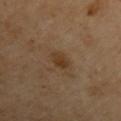No biopsy was performed on this lesion — it was imaged during a full skin examination and was not determined to be concerning. This image is a 15 mm lesion crop taken from a total-body photograph. A male patient, aged 68–72. The lesion's longest dimension is about 2.5 mm. The total-body-photography lesion software estimated an average lesion color of about L≈35 a*≈15 b*≈30 (CIELAB), about 7 CIELAB-L* units darker than the surrounding skin, and a lesion-to-skin contrast of about 7 (normalized; higher = more distinct). And it measured a classifier nevus-likeness of about 40/100 and lesion-presence confidence of about 100/100. The tile uses cross-polarized illumination. On the left upper arm.